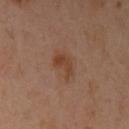The lesion was tiled from a total-body skin photograph and was not biopsied.